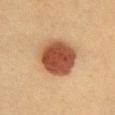Recorded during total-body skin imaging; not selected for excision or biopsy. A female subject aged approximately 40. Imaged with cross-polarized lighting. Automated image analysis of the tile measured a shape-asymmetry score of about 0.1 (0 = symmetric). The analysis additionally found a normalized border contrast of about 12. And it measured a border-irregularity rating of about 1/10, a within-lesion color-variation index near 5/10, and a peripheral color-asymmetry measure near 1.5. It also reported a classifier nevus-likeness of about 100/100. A 15 mm crop from a total-body photograph taken for skin-cancer surveillance. Longest diameter approximately 5 mm. On the chest.